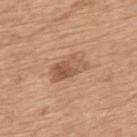biopsy status = no biopsy performed (imaged during a skin exam) | automated metrics = a lesion area of about 8.5 mm²; a border-irregularity index near 2.5/10 and a color-variation rating of about 6/10; an automated nevus-likeness rating near 20 out of 100 and a lesion-detection confidence of about 100/100 | image source = 15 mm crop, total-body photography | site = the upper back | subject = male, about 80 years old | illumination = white-light | size = ≈4.5 mm.